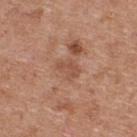Impression:
Recorded during total-body skin imaging; not selected for excision or biopsy.
Clinical summary:
A close-up tile cropped from a whole-body skin photograph, about 15 mm across. On the upper back. The lesion's longest dimension is about 3 mm. A female subject, in their 40s. The tile uses white-light illumination.